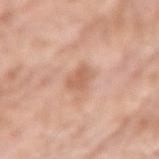Imaged during a routine full-body skin examination; the lesion was not biopsied and no histopathology is available.